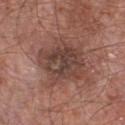Impression:
Recorded during total-body skin imaging; not selected for excision or biopsy.
Context:
The patient is a male about 65 years old. From the chest. Longest diameter approximately 5.5 mm. Captured under white-light illumination. Cropped from a total-body skin-imaging series; the visible field is about 15 mm.